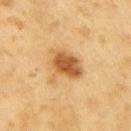The lesion was tiled from a total-body skin photograph and was not biopsied. Located on the arm. This image is a 15 mm lesion crop taken from a total-body photograph. A male subject, aged approximately 60.